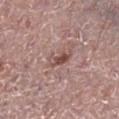| key | value |
|---|---|
| notes | imaged on a skin check; not biopsied |